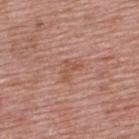| feature | finding |
|---|---|
| follow-up | total-body-photography surveillance lesion; no biopsy |
| diameter | ~3 mm (longest diameter) |
| subject | male, approximately 50 years of age |
| lighting | white-light |
| body site | the upper back |
| acquisition | 15 mm crop, total-body photography |